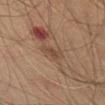{
  "biopsy_status": "not biopsied; imaged during a skin examination",
  "image": {
    "source": "total-body photography crop",
    "field_of_view_mm": 15
  },
  "automated_metrics": {
    "cielab_L": 40,
    "cielab_a": 15,
    "cielab_b": 26,
    "vs_skin_darker_L": 6.0,
    "vs_skin_contrast_norm": 5.5,
    "border_irregularity_0_10": 4.0,
    "color_variation_0_10": 1.0,
    "peripheral_color_asymmetry": 0.5,
    "nevus_likeness_0_100": 0,
    "lesion_detection_confidence_0_100": 95
  },
  "patient": {
    "sex": "male",
    "age_approx": 60
  },
  "lesion_size": {
    "long_diameter_mm_approx": 3.0
  },
  "site": "back",
  "lighting": "cross-polarized"
}